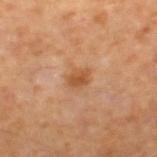No biopsy was performed on this lesion — it was imaged during a full skin examination and was not determined to be concerning.